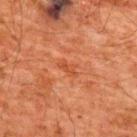notes = catalogued during a skin exam; not biopsied | acquisition = ~15 mm tile from a whole-body skin photo | subject = male, roughly 60 years of age | anatomic site = the upper back.